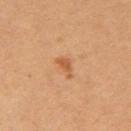Impression:
This lesion was catalogued during total-body skin photography and was not selected for biopsy.
Context:
This is a cross-polarized tile. The lesion is located on the right upper arm. A female subject in their 30s. A 15 mm close-up tile from a total-body photography series done for melanoma screening. Longest diameter approximately 2.5 mm.